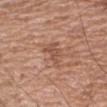{
  "biopsy_status": "not biopsied; imaged during a skin examination",
  "image": {
    "source": "total-body photography crop",
    "field_of_view_mm": 15
  },
  "site": "right upper arm",
  "lesion_size": {
    "long_diameter_mm_approx": 3.0
  },
  "automated_metrics": {
    "area_mm2_approx": 5.5,
    "eccentricity": 0.65,
    "shape_asymmetry": 0.5,
    "cielab_L": 52,
    "cielab_a": 22,
    "cielab_b": 29,
    "vs_skin_darker_L": 8.0,
    "vs_skin_contrast_norm": 6.0,
    "nevus_likeness_0_100": 0,
    "lesion_detection_confidence_0_100": 100
  },
  "lighting": "white-light",
  "patient": {
    "sex": "male",
    "age_approx": 65
  }
}